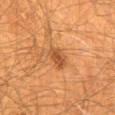biopsy status: catalogued during a skin exam; not biopsied | image-analysis metrics: a shape-asymmetry score of about 0.2 (0 = symmetric) | location: the mid back | subject: male, aged approximately 65 | lesion diameter: ~3 mm (longest diameter) | image source: 15 mm crop, total-body photography.